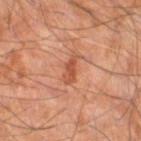notes = no biopsy performed (imaged during a skin exam) | automated lesion analysis = a footprint of about 4 mm²; an automated nevus-likeness rating near 0 out of 100 and lesion-presence confidence of about 100/100 | tile lighting = cross-polarized | patient = male, about 55 years old | image = ~15 mm crop, total-body skin-cancer survey | location = the right thigh.